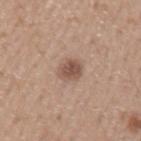biopsy_status: not biopsied; imaged during a skin examination
image:
  source: total-body photography crop
  field_of_view_mm: 15
lesion_size:
  long_diameter_mm_approx: 3.0
site: left upper arm
automated_metrics:
  cielab_L: 52
  cielab_a: 19
  cielab_b: 26
  vs_skin_darker_L: 12.0
  vs_skin_contrast_norm: 8.0
  border_irregularity_0_10: 2.0
  color_variation_0_10: 2.5
  peripheral_color_asymmetry: 1.0
  nevus_likeness_0_100: 85
  lesion_detection_confidence_0_100: 100
patient:
  sex: male
  age_approx: 20